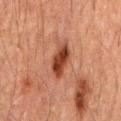{"lighting": "cross-polarized", "patient": {"sex": "male", "age_approx": 60}, "image": {"source": "total-body photography crop", "field_of_view_mm": 15}, "lesion_size": {"long_diameter_mm_approx": 4.5}, "site": "mid back"}